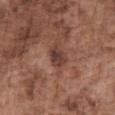{
  "biopsy_status": "not biopsied; imaged during a skin examination",
  "image": {
    "source": "total-body photography crop",
    "field_of_view_mm": 15
  },
  "site": "front of the torso",
  "automated_metrics": {
    "area_mm2_approx": 4.0,
    "shape_asymmetry": 0.2,
    "cielab_L": 39,
    "cielab_a": 20,
    "cielab_b": 24,
    "vs_skin_darker_L": 10.0
  },
  "lesion_size": {
    "long_diameter_mm_approx": 2.5
  },
  "patient": {
    "sex": "male",
    "age_approx": 75
  },
  "lighting": "white-light"
}